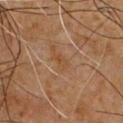Case summary:
* notes: catalogued during a skin exam; not biopsied
* acquisition: ~15 mm tile from a whole-body skin photo
* anatomic site: the chest
* automated lesion analysis: a footprint of about 3 mm²; a border-irregularity rating of about 2.5/10 and a within-lesion color-variation index near 2/10
* illumination: cross-polarized illumination
* diameter: ~2.5 mm (longest diameter)
* subject: male, aged 58–62